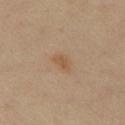workup = imaged on a skin check; not biopsied
location = the chest
image source = 15 mm crop, total-body photography
patient = male, aged 48–52